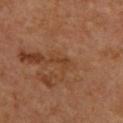notes — no biopsy performed (imaged during a skin exam); lighting — cross-polarized; subject — male, aged 58 to 62; site — the upper back; acquisition — ~15 mm tile from a whole-body skin photo; lesion diameter — about 2.5 mm.